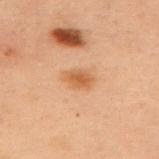This lesion was catalogued during total-body skin photography and was not selected for biopsy.
A 15 mm close-up extracted from a 3D total-body photography capture.
This is a cross-polarized tile.
A female patient, aged 53 to 57.
Longest diameter approximately 3.5 mm.
On the back.